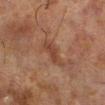A region of skin cropped from a whole-body photographic capture, roughly 15 mm wide. A male subject, roughly 70 years of age. From the right lower leg.